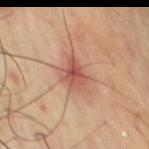Q: Was this lesion biopsied?
A: total-body-photography surveillance lesion; no biopsy
Q: Where on the body is the lesion?
A: the chest
Q: Illumination type?
A: cross-polarized
Q: Automated lesion metrics?
A: an area of roughly 8 mm², an eccentricity of roughly 0.65, and a shape-asymmetry score of about 0.3 (0 = symmetric); a within-lesion color-variation index near 5/10 and a peripheral color-asymmetry measure near 1.5
Q: What is the imaging modality?
A: ~15 mm crop, total-body skin-cancer survey
Q: What are the patient's age and sex?
A: male, in their 70s
Q: How large is the lesion?
A: ≈3.5 mm